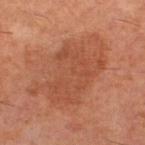The lesion was photographed on a routine skin check and not biopsied; there is no pathology result. The total-body-photography lesion software estimated a lesion area of about 35 mm² and a symmetry-axis asymmetry near 0.35. It also reported a lesion color around L≈46 a*≈25 b*≈32 in CIELAB, roughly 7 lightness units darker than nearby skin, and a lesion-to-skin contrast of about 5.5 (normalized; higher = more distinct). The analysis additionally found internal color variation of about 3 on a 0–10 scale and a peripheral color-asymmetry measure near 1. And it measured an automated nevus-likeness rating near 5 out of 100 and a detector confidence of about 100 out of 100 that the crop contains a lesion. A roughly 15 mm field-of-view crop from a total-body skin photograph. Approximately 9.5 mm at its widest. The subject is a male aged approximately 60. From the left lower leg. Imaged with cross-polarized lighting.